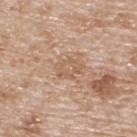Recorded during total-body skin imaging; not selected for excision or biopsy. A male subject, approximately 80 years of age. A 15 mm close-up extracted from a 3D total-body photography capture. On the upper back.